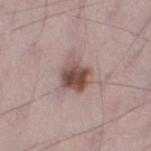The total-body-photography lesion software estimated an area of roughly 10 mm², an outline eccentricity of about 0.4 (0 = round, 1 = elongated), and a symmetry-axis asymmetry near 0.3. The analysis additionally found a mean CIELAB color near L≈50 a*≈18 b*≈21. And it measured a color-variation rating of about 8/10 and peripheral color asymmetry of about 3.
A male patient in their 70s.
The lesion is on the left thigh.
The tile uses white-light illumination.
A 15 mm close-up extracted from a 3D total-body photography capture.
Approximately 4 mm at its widest.